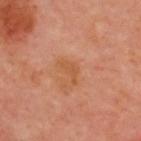follow-up: catalogued during a skin exam; not biopsied | image: ~15 mm tile from a whole-body skin photo | size: ~3 mm (longest diameter) | body site: the upper back | patient: male, aged 48–52 | lighting: cross-polarized.